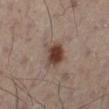A male subject aged approximately 50. Cropped from a total-body skin-imaging series; the visible field is about 15 mm. On the left leg.